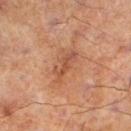Imaged during a routine full-body skin examination; the lesion was not biopsied and no histopathology is available. The tile uses cross-polarized illumination. A 15 mm crop from a total-body photograph taken for skin-cancer surveillance. An algorithmic analysis of the crop reported a footprint of about 6 mm² and a shape eccentricity near 0.9. The analysis additionally found a mean CIELAB color near L≈42 a*≈21 b*≈28, roughly 7 lightness units darker than nearby skin, and a lesion-to-skin contrast of about 6 (normalized; higher = more distinct). It also reported a border-irregularity rating of about 5/10, a within-lesion color-variation index near 2.5/10, and peripheral color asymmetry of about 0.5. From the left lower leg. About 4 mm across. A male patient, aged approximately 70.